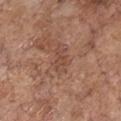Impression:
This lesion was catalogued during total-body skin photography and was not selected for biopsy.
Background:
The lesion is located on the front of the torso. The subject is a male aged 68 to 72. The total-body-photography lesion software estimated about 6 CIELAB-L* units darker than the surrounding skin. Imaged with white-light lighting. A region of skin cropped from a whole-body photographic capture, roughly 15 mm wide.